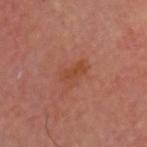<tbp_lesion>
<biopsy_status>not biopsied; imaged during a skin examination</biopsy_status>
<lighting>cross-polarized</lighting>
<patient>
  <sex>male</sex>
  <age_approx>65</age_approx>
</patient>
<site>head or neck</site>
<lesion_size>
  <long_diameter_mm_approx>3.0</long_diameter_mm_approx>
</lesion_size>
<automated_metrics>
  <area_mm2_approx>4.5</area_mm2_approx>
  <eccentricity>0.85</eccentricity>
  <shape_asymmetry>0.3</shape_asymmetry>
  <cielab_L>46</cielab_L>
  <cielab_a>27</cielab_a>
  <cielab_b>33</cielab_b>
  <vs_skin_darker_L>6.0</vs_skin_darker_L>
  <vs_skin_contrast_norm>6.0</vs_skin_contrast_norm>
  <border_irregularity_0_10>3.5</border_irregularity_0_10>
  <peripheral_color_asymmetry>1.0</peripheral_color_asymmetry>
</automated_metrics>
<image>
  <source>total-body photography crop</source>
  <field_of_view_mm>15</field_of_view_mm>
</image>
</tbp_lesion>